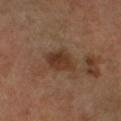follow-up: catalogued during a skin exam; not biopsied | patient: male, in their mid- to late 60s | site: the right lower leg | image-analysis metrics: a footprint of about 7.5 mm², an eccentricity of roughly 0.75, and a shape-asymmetry score of about 0.3 (0 = symmetric); a lesion–skin lightness drop of about 9 and a lesion-to-skin contrast of about 8.5 (normalized; higher = more distinct); a within-lesion color-variation index near 3/10; a nevus-likeness score of about 80/100 and lesion-presence confidence of about 100/100 | lesion diameter: ≈3.5 mm | image source: ~15 mm tile from a whole-body skin photo | illumination: cross-polarized.